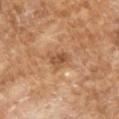Part of a total-body skin-imaging series; this lesion was reviewed on a skin check and was not flagged for biopsy.
A roughly 15 mm field-of-view crop from a total-body skin photograph.
The patient is a male aged around 65.